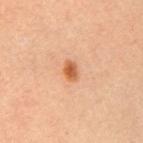Part of a total-body skin-imaging series; this lesion was reviewed on a skin check and was not flagged for biopsy. Located on the right upper arm. A female subject, aged 33–37. A lesion tile, about 15 mm wide, cut from a 3D total-body photograph. An algorithmic analysis of the crop reported an area of roughly 3 mm², an outline eccentricity of about 0.8 (0 = round, 1 = elongated), and a shape-asymmetry score of about 0.25 (0 = symmetric). And it measured a border-irregularity rating of about 2/10, internal color variation of about 3 on a 0–10 scale, and a peripheral color-asymmetry measure near 1. The software also gave a classifier nevus-likeness of about 100/100 and a detector confidence of about 100 out of 100 that the crop contains a lesion.